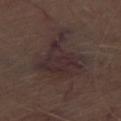Q: How was the tile lit?
A: white-light
Q: What is the imaging modality?
A: ~15 mm crop, total-body skin-cancer survey
Q: What is the lesion's diameter?
A: about 6.5 mm
Q: What is the anatomic site?
A: the leg
Q: What did automated image analysis measure?
A: a lesion color around L≈28 a*≈14 b*≈14 in CIELAB and a normalized border contrast of about 7.5; a classifier nevus-likeness of about 0/100 and lesion-presence confidence of about 85/100
Q: Patient demographics?
A: male, roughly 70 years of age